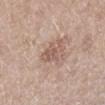Clinical impression:
Recorded during total-body skin imaging; not selected for excision or biopsy.
Context:
The lesion's longest dimension is about 3 mm. The lesion-visualizer software estimated a footprint of about 6.5 mm², a shape eccentricity near 0.45, and a symmetry-axis asymmetry near 0.4. And it measured an average lesion color of about L≈57 a*≈18 b*≈25 (CIELAB), a lesion–skin lightness drop of about 9, and a lesion-to-skin contrast of about 6 (normalized; higher = more distinct). And it measured a border-irregularity rating of about 5/10 and a within-lesion color-variation index near 2/10. And it measured a lesion-detection confidence of about 100/100. Imaged with white-light lighting. Located on the right lower leg. A 15 mm close-up tile from a total-body photography series done for melanoma screening. The patient is a female roughly 55 years of age.